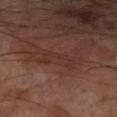Clinical impression: Captured during whole-body skin photography for melanoma surveillance; the lesion was not biopsied. Clinical summary: From the left lower leg. Cropped from a total-body skin-imaging series; the visible field is about 15 mm. The patient is a male aged around 70.